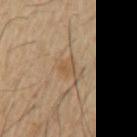<record>
<biopsy_status>not biopsied; imaged during a skin examination</biopsy_status>
<lighting>cross-polarized</lighting>
<site>left upper arm</site>
<image>
  <source>total-body photography crop</source>
  <field_of_view_mm>15</field_of_view_mm>
</image>
<lesion_size>
  <long_diameter_mm_approx>2.5</long_diameter_mm_approx>
</lesion_size>
<patient>
  <sex>male</sex>
  <age_approx>60</age_approx>
</patient>
</record>